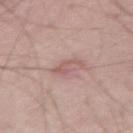Notes:
* follow-up — total-body-photography surveillance lesion; no biopsy
* imaging modality — ~15 mm crop, total-body skin-cancer survey
* subject — male, approximately 65 years of age
* anatomic site — the abdomen